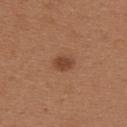notes: imaged on a skin check; not biopsied | anatomic site: the upper back | tile lighting: white-light illumination | patient: female, aged approximately 30 | image source: total-body-photography crop, ~15 mm field of view.